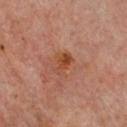| key | value |
|---|---|
| follow-up | total-body-photography surveillance lesion; no biopsy |
| anatomic site | the chest |
| tile lighting | cross-polarized |
| patient | female, aged 58–62 |
| image | ~15 mm tile from a whole-body skin photo |
| lesion size | ≈3 mm |